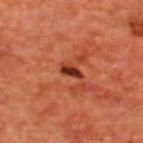Impression:
The lesion was photographed on a routine skin check and not biopsied; there is no pathology result.
Acquisition and patient details:
A 15 mm crop from a total-body photograph taken for skin-cancer surveillance. From the upper back. A male subject aged 63–67. The recorded lesion diameter is about 2.5 mm. Imaged with cross-polarized lighting.